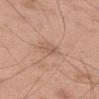workup = catalogued during a skin exam; not biopsied
lesion diameter = ≈2.5 mm
image source = 15 mm crop, total-body photography
subject = male, approximately 50 years of age
site = the leg